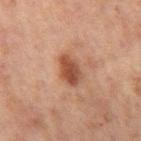Findings:
- biopsy status — imaged on a skin check; not biopsied